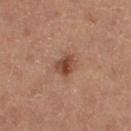Background:
Imaged with cross-polarized lighting. Approximately 3 mm at its widest. A 15 mm close-up tile from a total-body photography series done for melanoma screening. The subject is a male aged 53–57. Located on the left thigh. An algorithmic analysis of the crop reported an area of roughly 5 mm² and a shape eccentricity near 0.4. And it measured a lesion color around L≈44 a*≈22 b*≈28 in CIELAB, about 11 CIELAB-L* units darker than the surrounding skin, and a normalized border contrast of about 8.5.